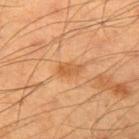tile lighting: cross-polarized
body site: the right upper arm
patient: male, about 55 years old
acquisition: total-body-photography crop, ~15 mm field of view
automated lesion analysis: roughly 8 lightness units darker than nearby skin and a lesion-to-skin contrast of about 6.5 (normalized; higher = more distinct); a within-lesion color-variation index near 1.5/10 and radial color variation of about 0.5
diameter: ≈3 mm